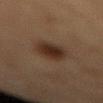Notes:
– notes: no biopsy performed (imaged during a skin exam)
– lighting: cross-polarized illumination
– automated lesion analysis: a lesion color around L≈25 a*≈14 b*≈22 in CIELAB, roughly 9 lightness units darker than nearby skin, and a normalized border contrast of about 10
– lesion diameter: ~4.5 mm (longest diameter)
– anatomic site: the mid back
– acquisition: ~15 mm crop, total-body skin-cancer survey
– patient: male, in their mid- to late 80s or older